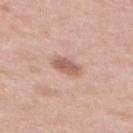Notes:
* anatomic site · the upper back
* image · 15 mm crop, total-body photography
* TBP lesion metrics · a footprint of about 5.5 mm² and a symmetry-axis asymmetry near 0.2; a border-irregularity rating of about 2/10, internal color variation of about 2.5 on a 0–10 scale, and radial color variation of about 1; a detector confidence of about 100 out of 100 that the crop contains a lesion
* subject · female, in their mid-60s
* tile lighting · white-light illumination
* lesion size · about 3 mm